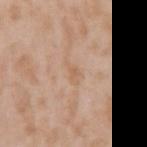The lesion was photographed on a routine skin check and not biopsied; there is no pathology result. The lesion-visualizer software estimated an outline eccentricity of about 0.85 (0 = round, 1 = elongated) and a shape-asymmetry score of about 0.45 (0 = symmetric). The software also gave border irregularity of about 5 on a 0–10 scale and internal color variation of about 0 on a 0–10 scale. And it measured a lesion-detection confidence of about 100/100. A roughly 15 mm field-of-view crop from a total-body skin photograph. Located on the left upper arm. The subject is a male approximately 45 years of age. The tile uses white-light illumination.